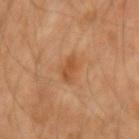workup: catalogued during a skin exam; not biopsied
size: ≈2.5 mm
patient: male, roughly 50 years of age
image source: 15 mm crop, total-body photography
lighting: cross-polarized
anatomic site: the right forearm